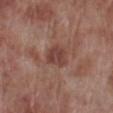No biopsy was performed on this lesion — it was imaged during a full skin examination and was not determined to be concerning.
The recorded lesion diameter is about 3 mm.
Located on the right lower leg.
The subject is a male in their 70s.
Captured under white-light illumination.
A region of skin cropped from a whole-body photographic capture, roughly 15 mm wide.